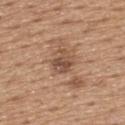biopsy_status: not biopsied; imaged during a skin examination
image:
  source: total-body photography crop
  field_of_view_mm: 15
lighting: white-light
site: upper back
lesion_size:
  long_diameter_mm_approx: 3.0
patient:
  sex: male
  age_approx: 65